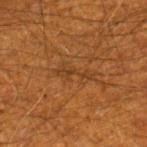Clinical impression:
Captured during whole-body skin photography for melanoma surveillance; the lesion was not biopsied.
Clinical summary:
From the right lower leg. A male patient, in their 60s. A roughly 15 mm field-of-view crop from a total-body skin photograph.